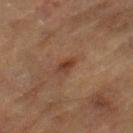Notes:
• workup: no biopsy performed (imaged during a skin exam)
• anatomic site: the left thigh
• lesion diameter: ≈2.5 mm
• image source: ~15 mm tile from a whole-body skin photo
• patient: male, in their mid- to late 80s or older